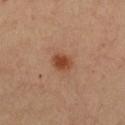Recorded during total-body skin imaging; not selected for excision or biopsy. Located on the chest. A female patient in their mid-40s. This is a cross-polarized tile. An algorithmic analysis of the crop reported an area of roughly 4.5 mm², a shape eccentricity near 0.6, and a shape-asymmetry score of about 0.15 (0 = symmetric). It also reported a border-irregularity rating of about 1.5/10 and peripheral color asymmetry of about 0.5. A 15 mm close-up tile from a total-body photography series done for melanoma screening.